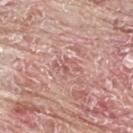Case summary:
* lighting · white-light illumination
* lesion diameter · about 3 mm
* patient · male, in their mid-60s
* acquisition · total-body-photography crop, ~15 mm field of view
* location · the upper back